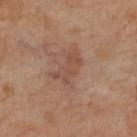This lesion was catalogued during total-body skin photography and was not selected for biopsy. The subject is a female aged around 50. The lesion is on the left lower leg. A 15 mm close-up extracted from a 3D total-body photography capture. Automated image analysis of the tile measured a footprint of about 7.5 mm² and a symmetry-axis asymmetry near 0.3. The analysis additionally found a border-irregularity index near 4/10 and a peripheral color-asymmetry measure near 1.